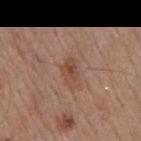Q: Was this lesion biopsied?
A: catalogued during a skin exam; not biopsied
Q: What lighting was used for the tile?
A: white-light
Q: Automated lesion metrics?
A: a normalized lesion–skin contrast near 6; a border-irregularity rating of about 3/10 and peripheral color asymmetry of about 1; a classifier nevus-likeness of about 5/100 and lesion-presence confidence of about 100/100
Q: Lesion size?
A: ≈3 mm
Q: Where on the body is the lesion?
A: the mid back
Q: How was this image acquired?
A: 15 mm crop, total-body photography
Q: What are the patient's age and sex?
A: male, aged 78 to 82